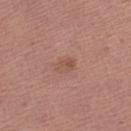Impression:
Part of a total-body skin-imaging series; this lesion was reviewed on a skin check and was not flagged for biopsy.
Image and clinical context:
Cropped from a whole-body photographic skin survey; the tile spans about 15 mm. A female subject, in their mid-60s. From the left lower leg.